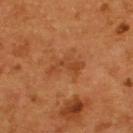follow-up: no biopsy performed (imaged during a skin exam).